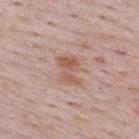Acquisition and patient details:
A close-up tile cropped from a whole-body skin photograph, about 15 mm across. The lesion is on the upper back. A female subject in their 50s.